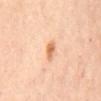No biopsy was performed on this lesion — it was imaged during a full skin examination and was not determined to be concerning.
A 15 mm crop from a total-body photograph taken for skin-cancer surveillance.
The patient is a female aged around 60.
About 2.5 mm across.
From the mid back.